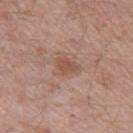Captured during whole-body skin photography for melanoma surveillance; the lesion was not biopsied. A 15 mm crop from a total-body photograph taken for skin-cancer surveillance. The lesion is located on the left thigh. A male subject in their mid-40s. The lesion's longest dimension is about 2.5 mm. Captured under white-light illumination.